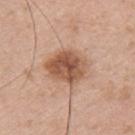Imaged during a routine full-body skin examination; the lesion was not biopsied and no histopathology is available.